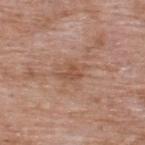No biopsy was performed on this lesion — it was imaged during a full skin examination and was not determined to be concerning. The lesion's longest dimension is about 3.5 mm. The patient is a male about 60 years old. This image is a 15 mm lesion crop taken from a total-body photograph. Imaged with white-light lighting. The lesion is on the upper back.